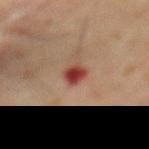<lesion>
  <biopsy_status>not biopsied; imaged during a skin examination</biopsy_status>
  <site>front of the torso</site>
  <image>
    <source>total-body photography crop</source>
    <field_of_view_mm>15</field_of_view_mm>
  </image>
  <lesion_size>
    <long_diameter_mm_approx>2.5</long_diameter_mm_approx>
  </lesion_size>
  <patient>
    <sex>male</sex>
    <age_approx>60</age_approx>
  </patient>
  <lighting>cross-polarized</lighting>
</lesion>